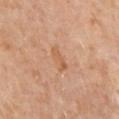Clinical impression: The lesion was tiled from a total-body skin photograph and was not biopsied. Background: Located on the left upper arm. A region of skin cropped from a whole-body photographic capture, roughly 15 mm wide. A female subject, aged around 50.